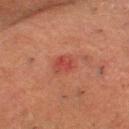The lesion was tiled from a total-body skin photograph and was not biopsied. A male subject, in their 80s. Located on the chest. A region of skin cropped from a whole-body photographic capture, roughly 15 mm wide.